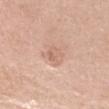Clinical impression:
The lesion was photographed on a routine skin check and not biopsied; there is no pathology result.
Clinical summary:
About 3 mm across. The patient is a female in their 40s. This image is a 15 mm lesion crop taken from a total-body photograph. On the head or neck. Captured under white-light illumination.